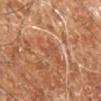No biopsy was performed on this lesion — it was imaged during a full skin examination and was not determined to be concerning.